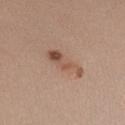biopsy status: no biopsy performed (imaged during a skin exam) | image source: total-body-photography crop, ~15 mm field of view | subject: female, in their 30s | anatomic site: the left forearm | diameter: about 5 mm | TBP lesion metrics: a footprint of about 7 mm² and two-axis asymmetry of about 0.4 | illumination: white-light illumination.